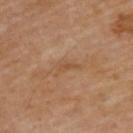lighting = cross-polarized illumination; patient = male, aged approximately 70; image source = ~15 mm crop, total-body skin-cancer survey; location = the back.